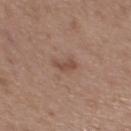Impression: Part of a total-body skin-imaging series; this lesion was reviewed on a skin check and was not flagged for biopsy. Background: Approximately 3 mm at its widest. Captured under white-light illumination. From the back. A roughly 15 mm field-of-view crop from a total-body skin photograph. The subject is a female about 50 years old.